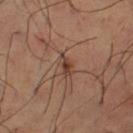The lesion was photographed on a routine skin check and not biopsied; there is no pathology result. From the right thigh. Cropped from a whole-body photographic skin survey; the tile spans about 15 mm. A male patient, aged around 65.